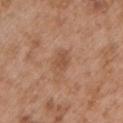This lesion was catalogued during total-body skin photography and was not selected for biopsy. The lesion is on the arm. The lesion's longest dimension is about 3 mm. This image is a 15 mm lesion crop taken from a total-body photograph. A male subject aged 53–57.